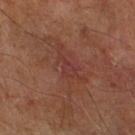Clinical impression:
This lesion was catalogued during total-body skin photography and was not selected for biopsy.
Clinical summary:
Measured at roughly 3 mm in maximum diameter. An algorithmic analysis of the crop reported an area of roughly 3.5 mm², a shape eccentricity near 0.85, and a shape-asymmetry score of about 0.5 (0 = symmetric). The software also gave a mean CIELAB color near L≈34 a*≈24 b*≈22, a lesion–skin lightness drop of about 5, and a lesion-to-skin contrast of about 5 (normalized; higher = more distinct). The analysis additionally found border irregularity of about 5 on a 0–10 scale and a color-variation rating of about 1/10. The software also gave a classifier nevus-likeness of about 0/100 and a lesion-detection confidence of about 95/100. A male patient, about 60 years old. A 15 mm close-up extracted from a 3D total-body photography capture. The lesion is located on the right leg. Imaged with cross-polarized lighting.